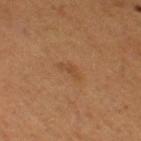Findings:
• workup — imaged on a skin check; not biopsied
• acquisition — ~15 mm crop, total-body skin-cancer survey
• location — the right thigh
• patient — female, about 55 years old